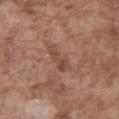| feature | finding |
|---|---|
| biopsy status | total-body-photography surveillance lesion; no biopsy |
| body site | the abdomen |
| size | ~4 mm (longest diameter) |
| acquisition | ~15 mm tile from a whole-body skin photo |
| patient | male, in their mid- to late 70s |
| illumination | white-light illumination |